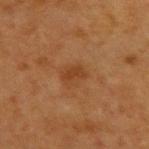Notes:
* image · total-body-photography crop, ~15 mm field of view
* location · the upper back
* subject · female, approximately 40 years of age